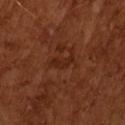workup: catalogued during a skin exam; not biopsied
subject: male, aged approximately 65
size: ≈3 mm
imaging modality: 15 mm crop, total-body photography
lighting: cross-polarized illumination
automated lesion analysis: roughly 6 lightness units darker than nearby skin and a normalized lesion–skin contrast near 6; border irregularity of about 6 on a 0–10 scale and a peripheral color-asymmetry measure near 0; lesion-presence confidence of about 100/100